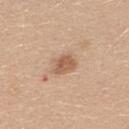Q: Was this lesion biopsied?
A: no biopsy performed (imaged during a skin exam)
Q: Patient demographics?
A: female, aged 23 to 27
Q: Illumination type?
A: white-light
Q: Lesion size?
A: ≈3 mm
Q: Where on the body is the lesion?
A: the upper back
Q: How was this image acquired?
A: ~15 mm tile from a whole-body skin photo